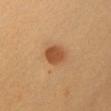biopsy status = no biopsy performed (imaged during a skin exam); location = the chest; imaging modality = total-body-photography crop, ~15 mm field of view; subject = female, roughly 35 years of age.